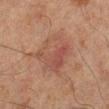| key | value |
|---|---|
| notes | no biopsy performed (imaged during a skin exam) |
| body site | the right lower leg |
| patient | male, aged 63 to 67 |
| lesion size | ≈5.5 mm |
| acquisition | total-body-photography crop, ~15 mm field of view |
| lighting | cross-polarized |